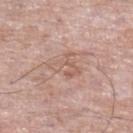Impression: No biopsy was performed on this lesion — it was imaged during a full skin examination and was not determined to be concerning. Image and clinical context: The subject is a male aged 73–77. The lesion-visualizer software estimated an average lesion color of about L≈59 a*≈19 b*≈26 (CIELAB) and a normalized lesion–skin contrast near 4.5. The lesion is on the left lower leg. A 15 mm crop from a total-body photograph taken for skin-cancer surveillance. The recorded lesion diameter is about 4 mm.